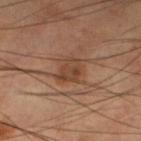The lesion was tiled from a total-body skin photograph and was not biopsied. Imaged with cross-polarized lighting. Cropped from a whole-body photographic skin survey; the tile spans about 15 mm. The lesion's longest dimension is about 3.5 mm. The subject is a male approximately 70 years of age. The lesion-visualizer software estimated a lesion area of about 6.5 mm², a shape eccentricity near 0.65, and a symmetry-axis asymmetry near 0.25. And it measured an average lesion color of about L≈41 a*≈19 b*≈30 (CIELAB), a lesion–skin lightness drop of about 7, and a normalized lesion–skin contrast near 6.5. It also reported a nevus-likeness score of about 0/100 and a detector confidence of about 100 out of 100 that the crop contains a lesion. The lesion is on the left lower leg.